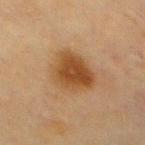biopsy status = no biopsy performed (imaged during a skin exam)
subject = female, approximately 55 years of age
imaging modality = total-body-photography crop, ~15 mm field of view
anatomic site = the chest